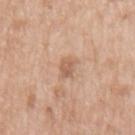Q: Was this lesion biopsied?
A: imaged on a skin check; not biopsied
Q: What kind of image is this?
A: ~15 mm crop, total-body skin-cancer survey
Q: What did automated image analysis measure?
A: a footprint of about 3.5 mm² and an outline eccentricity of about 0.8 (0 = round, 1 = elongated); internal color variation of about 1.5 on a 0–10 scale
Q: How large is the lesion?
A: ~2.5 mm (longest diameter)
Q: Where on the body is the lesion?
A: the arm
Q: Who is the patient?
A: male, about 50 years old